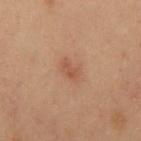{"biopsy_status": "not biopsied; imaged during a skin examination"}